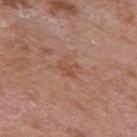automated metrics: a lesion-to-skin contrast of about 5.5 (normalized; higher = more distinct) | lesion diameter: ≈2.5 mm | image: ~15 mm crop, total-body skin-cancer survey | tile lighting: white-light illumination | anatomic site: the right upper arm | subject: male, aged 68–72.